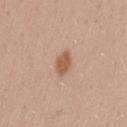Assessment: Recorded during total-body skin imaging; not selected for excision or biopsy. Image and clinical context: The lesion-visualizer software estimated a shape eccentricity near 0.75 and a shape-asymmetry score of about 0.25 (0 = symmetric). It also reported an average lesion color of about L≈57 a*≈21 b*≈32 (CIELAB), about 11 CIELAB-L* units darker than the surrounding skin, and a normalized border contrast of about 8. And it measured a nevus-likeness score of about 90/100 and a lesion-detection confidence of about 100/100. Located on the leg. Imaged with white-light lighting. A lesion tile, about 15 mm wide, cut from a 3D total-body photograph. The patient is a female roughly 30 years of age. The lesion's longest dimension is about 2.5 mm.